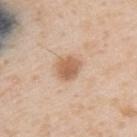workup — catalogued during a skin exam; not biopsied
patient — male, aged approximately 50
anatomic site — the back
diameter — ~3.5 mm (longest diameter)
imaging modality — total-body-photography crop, ~15 mm field of view